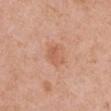<case>
<biopsy_status>not biopsied; imaged during a skin examination</biopsy_status>
<site>left upper arm</site>
<lighting>white-light</lighting>
<automated_metrics>
  <area_mm2_approx>6.0</area_mm2_approx>
  <eccentricity>0.75</eccentricity>
  <shape_asymmetry>0.2</shape_asymmetry>
  <cielab_L>60</cielab_L>
  <cielab_a>25</cielab_a>
  <cielab_b>33</cielab_b>
  <border_irregularity_0_10>2.0</border_irregularity_0_10>
  <color_variation_0_10>3.0</color_variation_0_10>
  <peripheral_color_asymmetry>1.0</peripheral_color_asymmetry>
  <nevus_likeness_0_100>15</nevus_likeness_0_100>
</automated_metrics>
<patient>
  <sex>female</sex>
  <age_approx>50</age_approx>
</patient>
<image>
  <source>total-body photography crop</source>
  <field_of_view_mm>15</field_of_view_mm>
</image>
<lesion_size>
  <long_diameter_mm_approx>3.0</long_diameter_mm_approx>
</lesion_size>
</case>